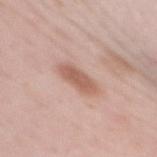– biopsy status · imaged on a skin check; not biopsied
– body site · the mid back
– acquisition · 15 mm crop, total-body photography
– subject · female, aged 38 to 42
– lesion size · about 5 mm
– illumination · white-light illumination
– image-analysis metrics · a footprint of about 6.5 mm², an eccentricity of roughly 0.95, and a symmetry-axis asymmetry near 0.2; a border-irregularity rating of about 3/10, internal color variation of about 2 on a 0–10 scale, and peripheral color asymmetry of about 0.5